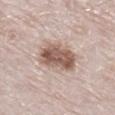{"biopsy_status": "not biopsied; imaged during a skin examination", "site": "right lower leg", "patient": {"sex": "male", "age_approx": 80}, "image": {"source": "total-body photography crop", "field_of_view_mm": 15}, "lesion_size": {"long_diameter_mm_approx": 5.5}, "automated_metrics": {"color_variation_0_10": 6.5, "peripheral_color_asymmetry": 2.5}}